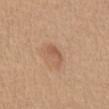This lesion was catalogued during total-body skin photography and was not selected for biopsy.
Longest diameter approximately 3 mm.
From the chest.
Automated image analysis of the tile measured a lesion area of about 4.5 mm², an outline eccentricity of about 0.85 (0 = round, 1 = elongated), and a shape-asymmetry score of about 0.4 (0 = symmetric). And it measured a classifier nevus-likeness of about 45/100 and lesion-presence confidence of about 100/100.
A lesion tile, about 15 mm wide, cut from a 3D total-body photograph.
The tile uses white-light illumination.
A female patient roughly 35 years of age.